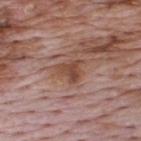Findings:
* location · the upper back
* image-analysis metrics · a lesion color around L≈47 a*≈21 b*≈28 in CIELAB, a lesion–skin lightness drop of about 9, and a normalized border contrast of about 7.5
* size · about 3.5 mm
* tile lighting · white-light illumination
* acquisition · total-body-photography crop, ~15 mm field of view
* patient · male, roughly 70 years of age